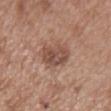Impression:
No biopsy was performed on this lesion — it was imaged during a full skin examination and was not determined to be concerning.
Context:
The lesion is on the right upper arm. A female patient, aged around 40. Approximately 4.5 mm at its widest. A 15 mm close-up tile from a total-body photography series done for melanoma screening. Imaged with white-light lighting.